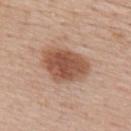From the upper back.
A 15 mm close-up tile from a total-body photography series done for melanoma screening.
A female patient, approximately 45 years of age.
Imaged with white-light lighting.
The recorded lesion diameter is about 5.5 mm.